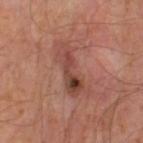Imaged during a routine full-body skin examination; the lesion was not biopsied and no histopathology is available.
The total-body-photography lesion software estimated a shape eccentricity near 0.95 and two-axis asymmetry of about 0.3. And it measured a classifier nevus-likeness of about 10/100 and a lesion-detection confidence of about 100/100.
A male subject, about 65 years old.
The lesion is on the left thigh.
Approximately 6.5 mm at its widest.
A roughly 15 mm field-of-view crop from a total-body skin photograph.